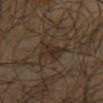| field | value |
|---|---|
| workup | no biopsy performed (imaged during a skin exam) |
| tile lighting | cross-polarized illumination |
| diameter | ≈3.5 mm |
| patient | male, aged approximately 65 |
| site | the mid back |
| image source | 15 mm crop, total-body photography |